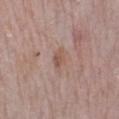Findings:
- notes · no biopsy performed (imaged during a skin exam)
- anatomic site · the left lower leg
- patient · female, aged 63 to 67
- image · ~15 mm crop, total-body skin-cancer survey